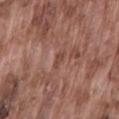• location · the lower back
• patient · male, approximately 75 years of age
• illumination · white-light illumination
• image source · ~15 mm crop, total-body skin-cancer survey
• lesion diameter · ~2.5 mm (longest diameter)